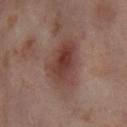Recorded during total-body skin imaging; not selected for excision or biopsy. A female patient aged 53–57. Imaged with cross-polarized lighting. On the right thigh. Cropped from a whole-body photographic skin survey; the tile spans about 15 mm.